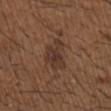{
  "biopsy_status": "not biopsied; imaged during a skin examination",
  "image": {
    "source": "total-body photography crop",
    "field_of_view_mm": 15
  },
  "lesion_size": {
    "long_diameter_mm_approx": 4.5
  },
  "lighting": "white-light",
  "patient": {
    "sex": "male",
    "age_approx": 50
  },
  "site": "right upper arm"
}